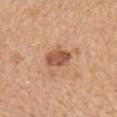No biopsy was performed on this lesion — it was imaged during a full skin examination and was not determined to be concerning.
A 15 mm close-up extracted from a 3D total-body photography capture.
The lesion's longest dimension is about 3.5 mm.
The patient is a female aged around 55.
From the upper back.